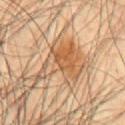Clinical impression:
The lesion was photographed on a routine skin check and not biopsied; there is no pathology result.
Background:
The lesion is on the chest. A 15 mm close-up tile from a total-body photography series done for melanoma screening. A male patient aged around 60.